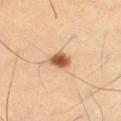Part of a total-body skin-imaging series; this lesion was reviewed on a skin check and was not flagged for biopsy. On the right thigh. Measured at roughly 3 mm in maximum diameter. Automated image analysis of the tile measured an eccentricity of roughly 0.75 and a shape-asymmetry score of about 0.25 (0 = symmetric). The software also gave a mean CIELAB color near L≈55 a*≈22 b*≈37, roughly 17 lightness units darker than nearby skin, and a normalized lesion–skin contrast near 11.5. The software also gave a border-irregularity index near 2/10, a within-lesion color-variation index near 3.5/10, and peripheral color asymmetry of about 1. And it measured lesion-presence confidence of about 100/100. A 15 mm crop from a total-body photograph taken for skin-cancer surveillance. Captured under cross-polarized illumination. The subject is a male aged approximately 55.